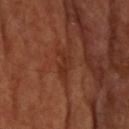Impression:
Captured during whole-body skin photography for melanoma surveillance; the lesion was not biopsied.
Background:
A roughly 15 mm field-of-view crop from a total-body skin photograph. From the head or neck.